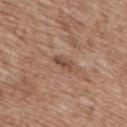subject = male, aged 68 to 72 | image = total-body-photography crop, ~15 mm field of view | body site = the upper back | size = ≈2.5 mm | automated lesion analysis = a lesion area of about 3 mm², a shape eccentricity near 0.9, and a symmetry-axis asymmetry near 0.3; a peripheral color-asymmetry measure near 0; an automated nevus-likeness rating near 0 out of 100 and lesion-presence confidence of about 90/100 | illumination = white-light illumination.